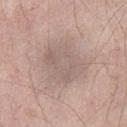Q: Was a biopsy performed?
A: total-body-photography surveillance lesion; no biopsy
Q: Patient demographics?
A: male, aged 53–57
Q: How large is the lesion?
A: about 6.5 mm
Q: What is the imaging modality?
A: ~15 mm crop, total-body skin-cancer survey
Q: Lesion location?
A: the left thigh
Q: What lighting was used for the tile?
A: white-light